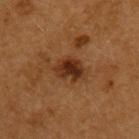{"biopsy_status": "not biopsied; imaged during a skin examination"}